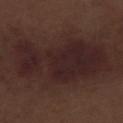location: the right lower leg | image-analysis metrics: an area of roughly 50 mm², a shape eccentricity near 0.9, and a shape-asymmetry score of about 0.25 (0 = symmetric); a normalized lesion–skin contrast near 9.5; a nevus-likeness score of about 25/100 | image source: 15 mm crop, total-body photography | lighting: white-light | patient: male, aged 68–72 | lesion diameter: ≈12 mm.